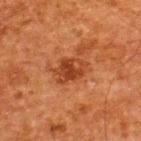Clinical impression: Recorded during total-body skin imaging; not selected for excision or biopsy. Image and clinical context: Automated image analysis of the tile measured a lesion area of about 8 mm² and a shape-asymmetry score of about 0.25 (0 = symmetric). The analysis additionally found an automated nevus-likeness rating near 45 out of 100 and lesion-presence confidence of about 100/100. A male patient, aged approximately 60. A lesion tile, about 15 mm wide, cut from a 3D total-body photograph. Approximately 4 mm at its widest. Located on the upper back. The tile uses cross-polarized illumination.